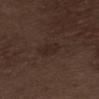Part of a total-body skin-imaging series; this lesion was reviewed on a skin check and was not flagged for biopsy.
Located on the left thigh.
A lesion tile, about 15 mm wide, cut from a 3D total-body photograph.
A male subject aged 68–72.